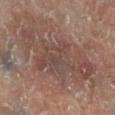{
  "biopsy_status": "not biopsied; imaged during a skin examination",
  "lighting": "cross-polarized",
  "image": {
    "source": "total-body photography crop",
    "field_of_view_mm": 15
  },
  "site": "right lower leg",
  "automated_metrics": {
    "eccentricity": 0.85,
    "cielab_L": 43,
    "cielab_a": 16,
    "cielab_b": 21,
    "vs_skin_darker_L": 7.0,
    "lesion_detection_confidence_0_100": 95
  },
  "patient": {
    "sex": "male",
    "age_approx": 65
  }
}